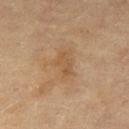Q: Is there a histopathology result?
A: catalogued during a skin exam; not biopsied
Q: Illumination type?
A: cross-polarized
Q: What is the anatomic site?
A: the leg
Q: How large is the lesion?
A: about 3 mm
Q: What are the patient's age and sex?
A: female, approximately 55 years of age
Q: What kind of image is this?
A: total-body-photography crop, ~15 mm field of view